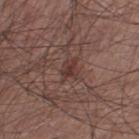| key | value |
|---|---|
| biopsy status | no biopsy performed (imaged during a skin exam) |
| site | the leg |
| patient | male, aged 53 to 57 |
| image | ~15 mm tile from a whole-body skin photo |